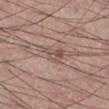Impression:
This lesion was catalogued during total-body skin photography and was not selected for biopsy.